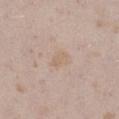Q: Was a biopsy performed?
A: total-body-photography surveillance lesion; no biopsy
Q: What kind of image is this?
A: ~15 mm tile from a whole-body skin photo
Q: Where on the body is the lesion?
A: the left thigh
Q: What are the patient's age and sex?
A: female, in their mid- to late 20s
Q: How was the tile lit?
A: white-light illumination